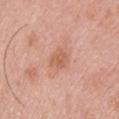Findings:
* notes — no biopsy performed (imaged during a skin exam)
* patient — male, approximately 25 years of age
* illumination — white-light illumination
* body site — the back
* automated lesion analysis — border irregularity of about 2 on a 0–10 scale, internal color variation of about 2.5 on a 0–10 scale, and peripheral color asymmetry of about 1
* image source — ~15 mm tile from a whole-body skin photo
* diameter — ~3 mm (longest diameter)